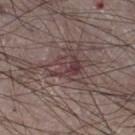Imaged during a routine full-body skin examination; the lesion was not biopsied and no histopathology is available.
The lesion's longest dimension is about 5 mm.
A male subject in their mid-70s.
Located on the leg.
A region of skin cropped from a whole-body photographic capture, roughly 15 mm wide.